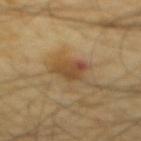{
  "biopsy_status": "not biopsied; imaged during a skin examination",
  "lesion_size": {
    "long_diameter_mm_approx": 3.5
  },
  "image": {
    "source": "total-body photography crop",
    "field_of_view_mm": 15
  },
  "patient": {
    "sex": "male",
    "age_approx": 65
  },
  "site": "mid back",
  "lighting": "cross-polarized"
}